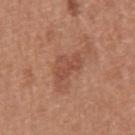No biopsy was performed on this lesion — it was imaged during a full skin examination and was not determined to be concerning. Imaged with white-light lighting. On the left upper arm. The patient is a female about 50 years old. The lesion-visualizer software estimated an area of roughly 5.5 mm², an outline eccentricity of about 0.85 (0 = round, 1 = elongated), and a symmetry-axis asymmetry near 0.45. Longest diameter approximately 4 mm. A 15 mm close-up extracted from a 3D total-body photography capture.